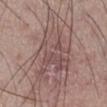Findings:
• workup: imaged on a skin check; not biopsied
• image: 15 mm crop, total-body photography
• patient: male, aged approximately 55
• site: the right lower leg